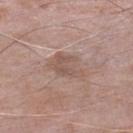{
  "biopsy_status": "not biopsied; imaged during a skin examination",
  "image": {
    "source": "total-body photography crop",
    "field_of_view_mm": 15
  },
  "patient": {
    "sex": "male",
    "age_approx": 75
  },
  "site": "left lower leg"
}